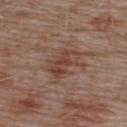biopsy status: total-body-photography surveillance lesion; no biopsy | tile lighting: white-light | patient: male, aged approximately 55 | lesion size: ~5 mm (longest diameter) | imaging modality: ~15 mm tile from a whole-body skin photo | anatomic site: the upper back.